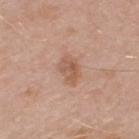Findings:
• follow-up · catalogued during a skin exam; not biopsied
• image source · 15 mm crop, total-body photography
• lesion size · ~3.5 mm (longest diameter)
• tile lighting · white-light illumination
• location · the left thigh
• subject · female, roughly 70 years of age